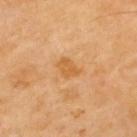This lesion was catalogued during total-body skin photography and was not selected for biopsy.
On the upper back.
About 2.5 mm across.
Captured under cross-polarized illumination.
Cropped from a whole-body photographic skin survey; the tile spans about 15 mm.
The total-body-photography lesion software estimated a lesion area of about 4 mm², an outline eccentricity of about 0.7 (0 = round, 1 = elongated), and two-axis asymmetry of about 0.3. The analysis additionally found an average lesion color of about L≈58 a*≈23 b*≈45 (CIELAB), roughly 8 lightness units darker than nearby skin, and a normalized border contrast of about 6.5. The software also gave a peripheral color-asymmetry measure near 0.5. And it measured a nevus-likeness score of about 0/100 and lesion-presence confidence of about 100/100.
The subject is a male roughly 70 years of age.